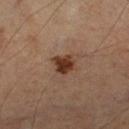Clinical impression:
Part of a total-body skin-imaging series; this lesion was reviewed on a skin check and was not flagged for biopsy.
Context:
Automated tile analysis of the lesion measured a footprint of about 4.5 mm², an outline eccentricity of about 0.3 (0 = round, 1 = elongated), and a shape-asymmetry score of about 0.35 (0 = symmetric). The software also gave an average lesion color of about L≈33 a*≈20 b*≈28 (CIELAB), a lesion–skin lightness drop of about 13, and a lesion-to-skin contrast of about 12 (normalized; higher = more distinct). The software also gave a border-irregularity index near 3/10 and a color-variation rating of about 2/10. The analysis additionally found a lesion-detection confidence of about 100/100. A region of skin cropped from a whole-body photographic capture, roughly 15 mm wide. The patient is a male about 65 years old. The lesion's longest dimension is about 2.5 mm. On the right thigh.